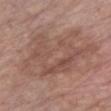Q: What kind of image is this?
A: ~15 mm tile from a whole-body skin photo
Q: Patient demographics?
A: male, roughly 75 years of age
Q: What did automated image analysis measure?
A: border irregularity of about 7 on a 0–10 scale, a color-variation rating of about 4.5/10, and radial color variation of about 1.5; a nevus-likeness score of about 0/100
Q: What is the anatomic site?
A: the chest